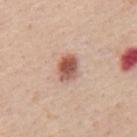<tbp_lesion>
<biopsy_status>not biopsied; imaged during a skin examination</biopsy_status>
<image>
  <source>total-body photography crop</source>
  <field_of_view_mm>15</field_of_view_mm>
</image>
<site>mid back</site>
<lesion_size>
  <long_diameter_mm_approx>3.0</long_diameter_mm_approx>
</lesion_size>
<patient>
  <sex>male</sex>
  <age_approx>65</age_approx>
</patient>
</tbp_lesion>